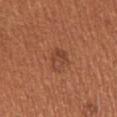follow-up = imaged on a skin check; not biopsied
subject = female, approximately 55 years of age
lesion diameter = ≈2.5 mm
image source = total-body-photography crop, ~15 mm field of view
anatomic site = the left upper arm
automated lesion analysis = an area of roughly 5 mm², a shape eccentricity near 0.35, and two-axis asymmetry of about 0.35; an average lesion color of about L≈44 a*≈25 b*≈32 (CIELAB) and a lesion–skin lightness drop of about 7
lighting = white-light